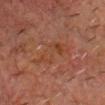Q: Is there a histopathology result?
A: imaged on a skin check; not biopsied
Q: What is the lesion's diameter?
A: ≈6.5 mm
Q: Patient demographics?
A: male, in their mid-60s
Q: What is the anatomic site?
A: the head or neck
Q: What lighting was used for the tile?
A: cross-polarized illumination
Q: What is the imaging modality?
A: 15 mm crop, total-body photography
Q: What did automated image analysis measure?
A: an average lesion color of about L≈33 a*≈20 b*≈26 (CIELAB); border irregularity of about 6.5 on a 0–10 scale, a within-lesion color-variation index near 4/10, and a peripheral color-asymmetry measure near 1; an automated nevus-likeness rating near 0 out of 100